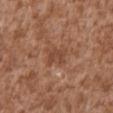Part of a total-body skin-imaging series; this lesion was reviewed on a skin check and was not flagged for biopsy. The patient is a male in their mid-40s. Automated tile analysis of the lesion measured a shape eccentricity near 0.4 and a symmetry-axis asymmetry near 0.2. The software also gave a mean CIELAB color near L≈45 a*≈22 b*≈30, about 7 CIELAB-L* units darker than the surrounding skin, and a normalized lesion–skin contrast near 5.5. Imaged with white-light lighting. Located on the abdomen. Cropped from a whole-body photographic skin survey; the tile spans about 15 mm.